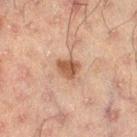biopsy_status: not biopsied; imaged during a skin examination
site: right thigh
lighting: cross-polarized
patient:
  sex: male
  age_approx: 50
image:
  source: total-body photography crop
  field_of_view_mm: 15
lesion_size:
  long_diameter_mm_approx: 2.5